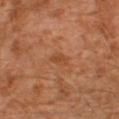biopsy_status: not biopsied; imaged during a skin examination
patient:
  sex: male
  age_approx: 30
lighting: cross-polarized
image:
  source: total-body photography crop
  field_of_view_mm: 15
site: left thigh
automated_metrics:
  area_mm2_approx: 3.0
  eccentricity: 0.85
  shape_asymmetry: 0.2
  nevus_likeness_0_100: 0